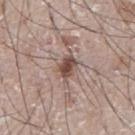workup = no biopsy performed (imaged during a skin exam)
image source = 15 mm crop, total-body photography
tile lighting = white-light
patient = male, aged 73 to 77
anatomic site = the abdomen
size = ~3 mm (longest diameter)
TBP lesion metrics = a lesion color around L≈48 a*≈18 b*≈22 in CIELAB and a normalized border contrast of about 9.5